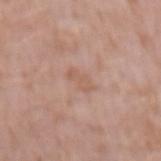| feature | finding |
|---|---|
| notes | total-body-photography surveillance lesion; no biopsy |
| lighting | white-light illumination |
| automated metrics | an eccentricity of roughly 0.85 and a shape-asymmetry score of about 0.3 (0 = symmetric); a border-irregularity rating of about 3.5/10, a color-variation rating of about 1/10, and a peripheral color-asymmetry measure near 0.5; an automated nevus-likeness rating near 0 out of 100 and a detector confidence of about 100 out of 100 that the crop contains a lesion |
| size | ~3 mm (longest diameter) |
| subject | male, aged 58 to 62 |
| image | ~15 mm crop, total-body skin-cancer survey |
| anatomic site | the arm |